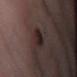Notes:
– biopsy status: total-body-photography surveillance lesion; no biopsy
– tile lighting: white-light illumination
– acquisition: 15 mm crop, total-body photography
– location: the leg
– diameter: about 3 mm
– subject: male, about 55 years old